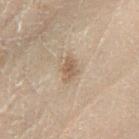Q: Was a biopsy performed?
A: no biopsy performed (imaged during a skin exam)
Q: Illumination type?
A: cross-polarized
Q: Patient demographics?
A: male, aged 68–72
Q: What is the anatomic site?
A: the left lower leg
Q: How was this image acquired?
A: ~15 mm tile from a whole-body skin photo
Q: Automated lesion metrics?
A: a lesion area of about 4 mm² and a shape eccentricity near 0.75; a mean CIELAB color near L≈43 a*≈11 b*≈23 and a lesion–skin lightness drop of about 7; a lesion-detection confidence of about 100/100
Q: How large is the lesion?
A: ~3 mm (longest diameter)